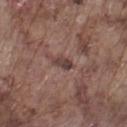Context: A close-up tile cropped from a whole-body skin photograph, about 15 mm across. A male subject, in their mid-70s. From the right lower leg. The tile uses white-light illumination.